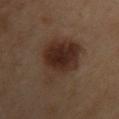{
  "biopsy_status": "not biopsied; imaged during a skin examination",
  "patient": {
    "sex": "female",
    "age_approx": 40
  },
  "image": {
    "source": "total-body photography crop",
    "field_of_view_mm": 15
  },
  "site": "upper back",
  "lighting": "cross-polarized"
}